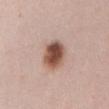Captured during whole-body skin photography for melanoma surveillance; the lesion was not biopsied. A close-up tile cropped from a whole-body skin photograph, about 15 mm across. Approximately 4 mm at its widest. Located on the abdomen. A female patient approximately 40 years of age.